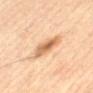follow-up: total-body-photography surveillance lesion; no biopsy
acquisition: total-body-photography crop, ~15 mm field of view
subject: male, approximately 70 years of age
body site: the front of the torso